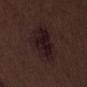Q: Is there a histopathology result?
A: no biopsy performed (imaged during a skin exam)
Q: Lesion location?
A: the leg
Q: Automated lesion metrics?
A: an area of roughly 22 mm², an outline eccentricity of about 0.75 (0 = round, 1 = elongated), and a symmetry-axis asymmetry near 0.25; a lesion color around L≈17 a*≈14 b*≈13 in CIELAB, roughly 6 lightness units darker than nearby skin, and a normalized lesion–skin contrast near 9.5; border irregularity of about 3 on a 0–10 scale, internal color variation of about 4.5 on a 0–10 scale, and radial color variation of about 1.5
Q: Who is the patient?
A: male, aged 68–72
Q: What is the imaging modality?
A: 15 mm crop, total-body photography
Q: Lesion size?
A: about 7 mm
Q: What lighting was used for the tile?
A: white-light illumination